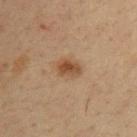This lesion was catalogued during total-body skin photography and was not selected for biopsy. The subject is a male about 35 years old. A close-up tile cropped from a whole-body skin photograph, about 15 mm across. This is a cross-polarized tile. Measured at roughly 3 mm in maximum diameter. Located on the chest. Automated image analysis of the tile measured a border-irregularity index near 2/10, a within-lesion color-variation index near 4.5/10, and peripheral color asymmetry of about 1.5. It also reported a classifier nevus-likeness of about 90/100 and a lesion-detection confidence of about 100/100.